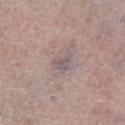subject: male, in their mid- to late 50s | diameter: about 2.5 mm | anatomic site: the left lower leg | image: total-body-photography crop, ~15 mm field of view | illumination: white-light illumination.